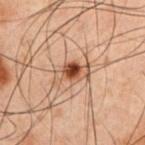The lesion was photographed on a routine skin check and not biopsied; there is no pathology result.
A close-up tile cropped from a whole-body skin photograph, about 15 mm across.
The lesion is located on the chest.
A male subject, aged 58 to 62.
Longest diameter approximately 4 mm.
Captured under cross-polarized illumination.